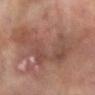Image and clinical context: The lesion's longest dimension is about 11 mm. The subject is a female in their 80s. From the arm. A 15 mm crop from a total-body photograph taken for skin-cancer surveillance. Captured under cross-polarized illumination.